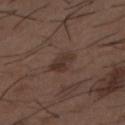No biopsy was performed on this lesion — it was imaged during a full skin examination and was not determined to be concerning. The recorded lesion diameter is about 3.5 mm. Automated tile analysis of the lesion measured a lesion area of about 6 mm², an eccentricity of roughly 0.8, and a shape-asymmetry score of about 0.2 (0 = symmetric). The software also gave a mean CIELAB color near L≈33 a*≈15 b*≈21, a lesion–skin lightness drop of about 7, and a normalized border contrast of about 7. The analysis additionally found a border-irregularity rating of about 2/10, a color-variation rating of about 3.5/10, and peripheral color asymmetry of about 1. The tile uses white-light illumination. A lesion tile, about 15 mm wide, cut from a 3D total-body photograph. A male subject, roughly 50 years of age. The lesion is located on the mid back.